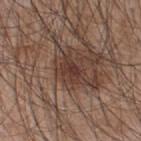biopsy status: total-body-photography surveillance lesion; no biopsy
image: 15 mm crop, total-body photography
lesion size: about 3 mm
lighting: white-light
subject: male, aged approximately 45
body site: the upper back